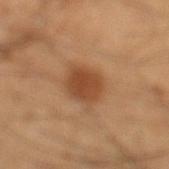image: total-body-photography crop, ~15 mm field of view; patient: male, approximately 40 years of age; diameter: ~4 mm (longest diameter); body site: the left lower leg; illumination: cross-polarized illumination.